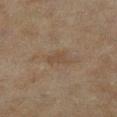The lesion is located on the left lower leg. A 15 mm close-up extracted from a 3D total-body photography capture. A female subject aged around 60. Automated tile analysis of the lesion measured a border-irregularity rating of about 3/10 and a within-lesion color-variation index near 1.5/10. The analysis additionally found a nevus-likeness score of about 0/100 and a lesion-detection confidence of about 100/100. The tile uses cross-polarized illumination. Longest diameter approximately 3 mm.